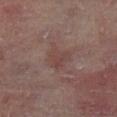Assessment: Captured during whole-body skin photography for melanoma surveillance; the lesion was not biopsied. Acquisition and patient details: Located on the left lower leg. A male subject aged 58 to 62. A 15 mm crop from a total-body photograph taken for skin-cancer surveillance. Longest diameter approximately 3.5 mm. The tile uses cross-polarized illumination.